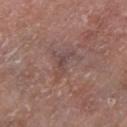From the left lower leg. Cropped from a whole-body photographic skin survey; the tile spans about 15 mm. The subject is a male aged approximately 80.